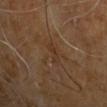The lesion was photographed on a routine skin check and not biopsied; there is no pathology result.
From the upper back.
A lesion tile, about 15 mm wide, cut from a 3D total-body photograph.
A male patient, aged approximately 60.
An algorithmic analysis of the crop reported a lesion area of about 3 mm² and an outline eccentricity of about 0.85 (0 = round, 1 = elongated). And it measured a lesion color around L≈31 a*≈15 b*≈27 in CIELAB, a lesion–skin lightness drop of about 4, and a normalized lesion–skin contrast near 4.5. It also reported a color-variation rating of about 0/10 and a peripheral color-asymmetry measure near 0.
This is a cross-polarized tile.